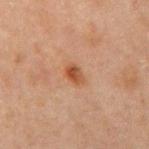Background:
The tile uses cross-polarized illumination. An algorithmic analysis of the crop reported about 10 CIELAB-L* units darker than the surrounding skin and a normalized lesion–skin contrast near 8.5. The software also gave a border-irregularity rating of about 2/10, internal color variation of about 4.5 on a 0–10 scale, and a peripheral color-asymmetry measure near 1.5. It also reported an automated nevus-likeness rating near 90 out of 100 and a detector confidence of about 100 out of 100 that the crop contains a lesion. A male patient, approximately 65 years of age. Approximately 2.5 mm at its widest. From the mid back. A 15 mm close-up tile from a total-body photography series done for melanoma screening.